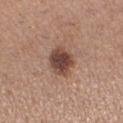Q: Was this lesion biopsied?
A: imaged on a skin check; not biopsied
Q: Patient demographics?
A: female, aged approximately 30
Q: What lighting was used for the tile?
A: white-light illumination
Q: How was this image acquired?
A: 15 mm crop, total-body photography
Q: What is the anatomic site?
A: the left lower leg